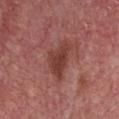No biopsy was performed on this lesion — it was imaged during a full skin examination and was not determined to be concerning. Cropped from a total-body skin-imaging series; the visible field is about 15 mm. A male subject, about 70 years old. Automated tile analysis of the lesion measured a border-irregularity index near 4/10 and internal color variation of about 3 on a 0–10 scale. It also reported a nevus-likeness score of about 5/100 and a lesion-detection confidence of about 100/100. Imaged with white-light lighting. Measured at roughly 5 mm in maximum diameter. From the chest.